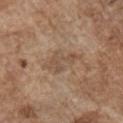notes = total-body-photography surveillance lesion; no biopsy
automated metrics = internal color variation of about 1 on a 0–10 scale and a peripheral color-asymmetry measure near 0.5; a classifier nevus-likeness of about 0/100
imaging modality = ~15 mm crop, total-body skin-cancer survey
tile lighting = white-light
patient = male, about 75 years old
body site = the chest
lesion diameter = ~4.5 mm (longest diameter)